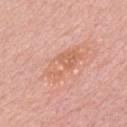Part of a total-body skin-imaging series; this lesion was reviewed on a skin check and was not flagged for biopsy. The lesion is located on the chest. The tile uses white-light illumination. About 5 mm across. A 15 mm close-up tile from a total-body photography series done for melanoma screening. A male subject in their mid-60s. Automated tile analysis of the lesion measured an area of roughly 11 mm². And it measured a mean CIELAB color near L≈64 a*≈24 b*≈33 and a normalized lesion–skin contrast near 5.5. The software also gave a border-irregularity index near 3/10, internal color variation of about 5.5 on a 0–10 scale, and a peripheral color-asymmetry measure near 2. The analysis additionally found an automated nevus-likeness rating near 0 out of 100.